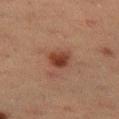notes: no biopsy performed (imaged during a skin exam) | automated lesion analysis: about 10 CIELAB-L* units darker than the surrounding skin and a lesion-to-skin contrast of about 9.5 (normalized; higher = more distinct) | subject: female, aged approximately 55 | tile lighting: cross-polarized illumination | site: the left thigh | acquisition: ~15 mm crop, total-body skin-cancer survey | diameter: about 2.5 mm.